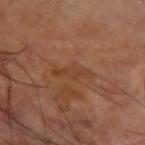<lesion>
  <biopsy_status>not biopsied; imaged during a skin examination</biopsy_status>
  <patient>
    <sex>male</sex>
    <age_approx>60</age_approx>
  </patient>
  <lighting>cross-polarized</lighting>
  <image>
    <source>total-body photography crop</source>
    <field_of_view_mm>15</field_of_view_mm>
  </image>
  <site>right forearm</site>
</lesion>